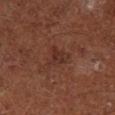notes = imaged on a skin check; not biopsied
imaging modality = 15 mm crop, total-body photography
subject = male, about 65 years old
diameter = ≈3.5 mm
TBP lesion metrics = a footprint of about 5.5 mm², an eccentricity of roughly 0.7, and two-axis asymmetry of about 0.55; an average lesion color of about L≈29 a*≈21 b*≈23 (CIELAB), a lesion–skin lightness drop of about 6, and a normalized lesion–skin contrast near 6; a nevus-likeness score of about 0/100
lighting = cross-polarized
anatomic site = the left lower leg